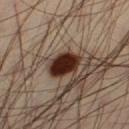| field | value |
|---|---|
| notes | imaged on a skin check; not biopsied |
| site | the left thigh |
| subject | male, aged around 40 |
| lesion size | ≈4 mm |
| imaging modality | total-body-photography crop, ~15 mm field of view |
| lighting | cross-polarized |